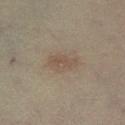Assessment:
Captured during whole-body skin photography for melanoma surveillance; the lesion was not biopsied.